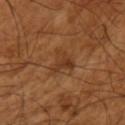The lesion was photographed on a routine skin check and not biopsied; there is no pathology result. A male patient aged 63–67. Measured at roughly 3.5 mm in maximum diameter. An algorithmic analysis of the crop reported a lesion area of about 6.5 mm², an outline eccentricity of about 0.7 (0 = round, 1 = elongated), and two-axis asymmetry of about 0.5. The software also gave a nevus-likeness score of about 0/100 and a lesion-detection confidence of about 100/100. The lesion is located on the left upper arm. Imaged with cross-polarized lighting. Cropped from a whole-body photographic skin survey; the tile spans about 15 mm.